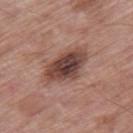follow-up: imaged on a skin check; not biopsied | image: ~15 mm crop, total-body skin-cancer survey | site: the right thigh | automated lesion analysis: a footprint of about 13 mm² and an outline eccentricity of about 0.8 (0 = round, 1 = elongated) | lighting: white-light illumination | patient: male, roughly 70 years of age | lesion size: ~5.5 mm (longest diameter).